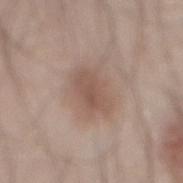biopsy status: catalogued during a skin exam; not biopsied | subject: male, roughly 45 years of age | image: ~15 mm tile from a whole-body skin photo | body site: the mid back | tile lighting: white-light illumination | automated lesion analysis: a footprint of about 9 mm², an outline eccentricity of about 0.8 (0 = round, 1 = elongated), and a symmetry-axis asymmetry near 0.2; a mean CIELAB color near L≈53 a*≈17 b*≈25 and a lesion–skin lightness drop of about 9; an automated nevus-likeness rating near 40 out of 100.